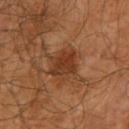follow-up — catalogued during a skin exam; not biopsied | illumination — cross-polarized illumination | location — the right upper arm | image-analysis metrics — border irregularity of about 3 on a 0–10 scale, internal color variation of about 4.5 on a 0–10 scale, and peripheral color asymmetry of about 1.5 | imaging modality — 15 mm crop, total-body photography | subject — male, aged approximately 65 | diameter — ≈4.5 mm.